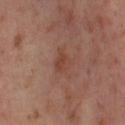biopsy status: no biopsy performed (imaged during a skin exam); subject: female, in their mid- to late 50s; image: 15 mm crop, total-body photography; lesion size: ≈3 mm; site: the right thigh; lighting: cross-polarized.